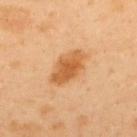The lesion was photographed on a routine skin check and not biopsied; there is no pathology result.
Cropped from a whole-body photographic skin survey; the tile spans about 15 mm.
About 5 mm across.
Imaged with cross-polarized lighting.
On the upper back.
Automated tile analysis of the lesion measured a lesion color around L≈60 a*≈25 b*≈44 in CIELAB and a lesion–skin lightness drop of about 12. The analysis additionally found border irregularity of about 2 on a 0–10 scale and a color-variation rating of about 3.5/10. The software also gave a nevus-likeness score of about 95/100.
A male patient, aged 38–42.